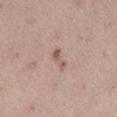Part of a total-body skin-imaging series; this lesion was reviewed on a skin check and was not flagged for biopsy. The lesion is on the right thigh. An algorithmic analysis of the crop reported lesion-presence confidence of about 100/100. The tile uses white-light illumination. A close-up tile cropped from a whole-body skin photograph, about 15 mm across. The patient is a female aged around 25. Longest diameter approximately 3.5 mm.